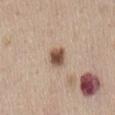biopsy status — imaged on a skin check; not biopsied | site — the back | size — ~2.5 mm (longest diameter) | illumination — white-light illumination | image — ~15 mm crop, total-body skin-cancer survey | subject — female, in their 50s.